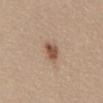Notes:
– notes · imaged on a skin check; not biopsied
– lesion size · about 3 mm
– subject · female, about 60 years old
– body site · the abdomen
– acquisition · ~15 mm crop, total-body skin-cancer survey
– automated metrics · a border-irregularity rating of about 2.5/10, a within-lesion color-variation index near 2.5/10, and peripheral color asymmetry of about 1; a classifier nevus-likeness of about 90/100 and a lesion-detection confidence of about 100/100